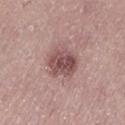Clinical impression: Imaged during a routine full-body skin examination; the lesion was not biopsied and no histopathology is available.